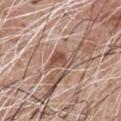| field | value |
|---|---|
| notes | no biopsy performed (imaged during a skin exam) |
| lesion diameter | ≈3.5 mm |
| illumination | white-light illumination |
| anatomic site | the chest |
| patient | male, aged approximately 60 |
| imaging modality | ~15 mm tile from a whole-body skin photo |
| automated metrics | a footprint of about 5.5 mm² and a shape eccentricity near 0.75; an automated nevus-likeness rating near 0 out of 100 and a detector confidence of about 95 out of 100 that the crop contains a lesion |